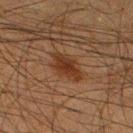biopsy status = no biopsy performed (imaged during a skin exam); patient = male, in their mid- to late 30s; size = about 4 mm; image-analysis metrics = an area of roughly 8 mm² and a shape eccentricity near 0.85; image source = ~15 mm tile from a whole-body skin photo; anatomic site = the right thigh.